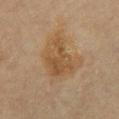biopsy status = no biopsy performed (imaged during a skin exam) | location = the chest | imaging modality = ~15 mm tile from a whole-body skin photo | subject = female, about 65 years old.